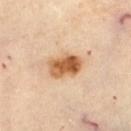Q: Is there a histopathology result?
A: total-body-photography surveillance lesion; no biopsy
Q: What is the imaging modality?
A: ~15 mm crop, total-body skin-cancer survey
Q: Lesion location?
A: the left thigh
Q: What is the lesion's diameter?
A: about 4 mm
Q: Illumination type?
A: cross-polarized
Q: What did automated image analysis measure?
A: a footprint of about 10 mm² and a symmetry-axis asymmetry near 0.2; a nevus-likeness score of about 100/100 and a lesion-detection confidence of about 100/100
Q: Who is the patient?
A: female, about 60 years old